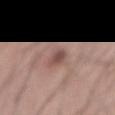workup = total-body-photography surveillance lesion; no biopsy | site = the abdomen | patient = male, approximately 55 years of age | illumination = white-light | lesion diameter = ≈3 mm | automated lesion analysis = a border-irregularity rating of about 2.5/10, a within-lesion color-variation index near 2/10, and a peripheral color-asymmetry measure near 0.5 | imaging modality = 15 mm crop, total-body photography.